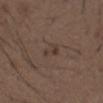Case summary:
– biopsy status: total-body-photography surveillance lesion; no biopsy
– image source: 15 mm crop, total-body photography
– image-analysis metrics: a lesion area of about 3 mm², an eccentricity of roughly 0.8, and two-axis asymmetry of about 0.45; border irregularity of about 4.5 on a 0–10 scale, a within-lesion color-variation index near 0.5/10, and radial color variation of about 0.5; a nevus-likeness score of about 0/100 and a lesion-detection confidence of about 100/100
– patient: male, aged around 50
– lighting: white-light
– anatomic site: the abdomen
– diameter: about 2.5 mm